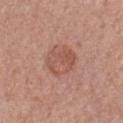Findings:
– biopsy status · total-body-photography surveillance lesion; no biopsy
– image · total-body-photography crop, ~15 mm field of view
– subject · female, aged approximately 60
– image-analysis metrics · a lesion area of about 10 mm², an outline eccentricity of about 0.4 (0 = round, 1 = elongated), and a symmetry-axis asymmetry near 0.15
– anatomic site · the left forearm